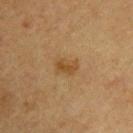This lesion was catalogued during total-body skin photography and was not selected for biopsy. This is a cross-polarized tile. A region of skin cropped from a whole-body photographic capture, roughly 15 mm wide. The recorded lesion diameter is about 3 mm. Located on the chest. A male patient aged 58–62.